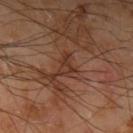{"biopsy_status": "not biopsied; imaged during a skin examination", "automated_metrics": {"border_irregularity_0_10": 7.0, "color_variation_0_10": 0.0, "peripheral_color_asymmetry": 0.0, "nevus_likeness_0_100": 0, "lesion_detection_confidence_0_100": 65}, "lesion_size": {"long_diameter_mm_approx": 3.0}, "site": "left upper arm", "lighting": "cross-polarized", "patient": {"sex": "male", "age_approx": 65}, "image": {"source": "total-body photography crop", "field_of_view_mm": 15}}